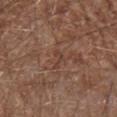The lesion was tiled from a total-body skin photograph and was not biopsied. Approximately 2.5 mm at its widest. The lesion is on the left forearm. A 15 mm close-up tile from a total-body photography series done for melanoma screening. A male patient about 70 years old. The lesion-visualizer software estimated an area of roughly 2 mm² and an outline eccentricity of about 0.9 (0 = round, 1 = elongated). This is a white-light tile.